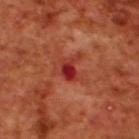<lesion>
  <biopsy_status>not biopsied; imaged during a skin examination</biopsy_status>
  <site>upper back</site>
  <image>
    <source>total-body photography crop</source>
    <field_of_view_mm>15</field_of_view_mm>
  </image>
  <patient>
    <sex>male</sex>
    <age_approx>70</age_approx>
  </patient>
  <lesion_size>
    <long_diameter_mm_approx>2.5</long_diameter_mm_approx>
  </lesion_size>
  <lighting>cross-polarized</lighting>
</lesion>